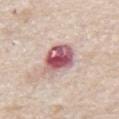The lesion is located on the front of the torso. Captured under white-light illumination. A 15 mm close-up extracted from a 3D total-body photography capture. A male patient approximately 65 years of age.